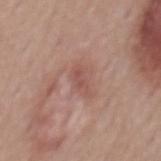Impression:
Imaged during a routine full-body skin examination; the lesion was not biopsied and no histopathology is available.
Context:
Automated tile analysis of the lesion measured a border-irregularity rating of about 5/10. A 15 mm close-up tile from a total-body photography series done for melanoma screening. A male patient, aged 53–57. The lesion is on the mid back.